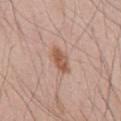Assessment: This lesion was catalogued during total-body skin photography and was not selected for biopsy. Background: On the mid back. Cropped from a whole-body photographic skin survey; the tile spans about 15 mm. Imaged with white-light lighting. Approximately 3.5 mm at its widest. The patient is a male in their mid-40s. Automated tile analysis of the lesion measured a classifier nevus-likeness of about 95/100.